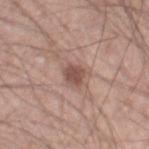Assessment: The lesion was tiled from a total-body skin photograph and was not biopsied. Context: The recorded lesion diameter is about 3 mm. The lesion-visualizer software estimated internal color variation of about 1.5 on a 0–10 scale and radial color variation of about 0.5. The software also gave a lesion-detection confidence of about 100/100. On the right thigh. Cropped from a total-body skin-imaging series; the visible field is about 15 mm. The patient is a male about 60 years old. Imaged with white-light lighting.